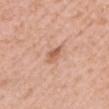Q: Is there a histopathology result?
A: imaged on a skin check; not biopsied
Q: Automated lesion metrics?
A: about 10 CIELAB-L* units darker than the surrounding skin and a normalized lesion–skin contrast near 7; a nevus-likeness score of about 5/100 and a detector confidence of about 100 out of 100 that the crop contains a lesion
Q: How large is the lesion?
A: about 2.5 mm
Q: How was the tile lit?
A: white-light
Q: What are the patient's age and sex?
A: female, about 40 years old
Q: What is the anatomic site?
A: the head or neck
Q: How was this image acquired?
A: ~15 mm tile from a whole-body skin photo